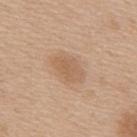Recorded during total-body skin imaging; not selected for excision or biopsy. The total-body-photography lesion software estimated a lesion area of about 8 mm², an outline eccentricity of about 0.8 (0 = round, 1 = elongated), and a shape-asymmetry score of about 0.2 (0 = symmetric). The analysis additionally found a border-irregularity index near 2/10 and radial color variation of about 0.5. A roughly 15 mm field-of-view crop from a total-body skin photograph. Longest diameter approximately 4 mm. A male subject about 60 years old. The lesion is on the mid back.